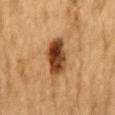Assessment:
Captured during whole-body skin photography for melanoma surveillance; the lesion was not biopsied.
Background:
A lesion tile, about 15 mm wide, cut from a 3D total-body photograph. The lesion is on the mid back. This is a cross-polarized tile. A male subject aged 83 or over. Longest diameter approximately 5 mm.